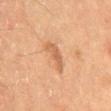biopsy_status: not biopsied; imaged during a skin examination
lighting: cross-polarized
patient:
  sex: male
  age_approx: 60
image:
  source: total-body photography crop
  field_of_view_mm: 15